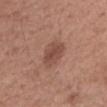Clinical impression:
Captured during whole-body skin photography for melanoma surveillance; the lesion was not biopsied.
Context:
A female subject aged 63–67. Measured at roughly 3.5 mm in maximum diameter. The total-body-photography lesion software estimated a lesion area of about 7.5 mm² and a symmetry-axis asymmetry near 0.25. The software also gave a mean CIELAB color near L≈48 a*≈22 b*≈26 and a lesion-to-skin contrast of about 7 (normalized; higher = more distinct). The software also gave a border-irregularity index near 2.5/10 and a color-variation rating of about 2.5/10. The software also gave an automated nevus-likeness rating near 70 out of 100 and a detector confidence of about 100 out of 100 that the crop contains a lesion. The lesion is located on the arm. Imaged with white-light lighting. A close-up tile cropped from a whole-body skin photograph, about 15 mm across.